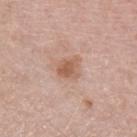Imaged during a routine full-body skin examination; the lesion was not biopsied and no histopathology is available. The lesion is on the arm. A 15 mm close-up extracted from a 3D total-body photography capture. A female subject, aged around 45.